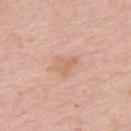| field | value |
|---|---|
| workup | catalogued during a skin exam; not biopsied |
| anatomic site | the right upper arm |
| image source | total-body-photography crop, ~15 mm field of view |
| subject | female, aged 63 to 67 |